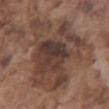The lesion was photographed on a routine skin check and not biopsied; there is no pathology result.
Located on the chest.
Cropped from a whole-body photographic skin survey; the tile spans about 15 mm.
A male patient, about 75 years old.